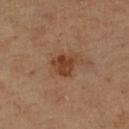Clinical impression: The lesion was tiled from a total-body skin photograph and was not biopsied. Background: Automated tile analysis of the lesion measured a lesion color around L≈42 a*≈22 b*≈33 in CIELAB, about 10 CIELAB-L* units darker than the surrounding skin, and a normalized lesion–skin contrast near 8.5. The software also gave a border-irregularity index near 3/10, a color-variation rating of about 3/10, and peripheral color asymmetry of about 1. The software also gave a classifier nevus-likeness of about 70/100 and a lesion-detection confidence of about 100/100. A close-up tile cropped from a whole-body skin photograph, about 15 mm across. Captured under cross-polarized illumination. The recorded lesion diameter is about 3 mm. On the leg. A female subject, aged 68–72.